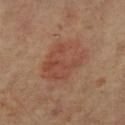The lesion was tiled from a total-body skin photograph and was not biopsied. Located on the left lower leg. The tile uses cross-polarized illumination. The recorded lesion diameter is about 5.5 mm. A region of skin cropped from a whole-body photographic capture, roughly 15 mm wide. An algorithmic analysis of the crop reported a border-irregularity index near 2.5/10, a color-variation rating of about 4.5/10, and radial color variation of about 1.5. The analysis additionally found a lesion-detection confidence of about 100/100. The patient is a female aged 68 to 72.